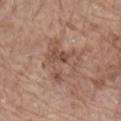• biopsy status: total-body-photography surveillance lesion; no biopsy
• patient: male, in their 80s
• tile lighting: white-light illumination
• acquisition: ~15 mm crop, total-body skin-cancer survey
• anatomic site: the lower back
• size: ≈5.5 mm